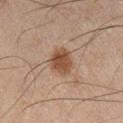The lesion was photographed on a routine skin check and not biopsied; there is no pathology result. Captured under cross-polarized illumination. A male patient, aged 43 to 47. An algorithmic analysis of the crop reported an outline eccentricity of about 0.5 (0 = round, 1 = elongated) and a shape-asymmetry score of about 0.15 (0 = symmetric). The analysis additionally found a lesion–skin lightness drop of about 10 and a normalized border contrast of about 9. And it measured a classifier nevus-likeness of about 95/100 and a detector confidence of about 100 out of 100 that the crop contains a lesion. Cropped from a total-body skin-imaging series; the visible field is about 15 mm. The lesion is located on the right lower leg.